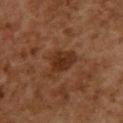Part of a total-body skin-imaging series; this lesion was reviewed on a skin check and was not flagged for biopsy. The recorded lesion diameter is about 4.5 mm. A region of skin cropped from a whole-body photographic capture, roughly 15 mm wide. An algorithmic analysis of the crop reported a border-irregularity index near 3.5/10, internal color variation of about 2.5 on a 0–10 scale, and peripheral color asymmetry of about 1. The analysis additionally found a detector confidence of about 100 out of 100 that the crop contains a lesion. A female patient, aged 58 to 62. On the upper back.